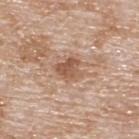Impression: Recorded during total-body skin imaging; not selected for excision or biopsy. Context: Located on the upper back. The subject is a male in their 80s. Automated image analysis of the tile measured border irregularity of about 3.5 on a 0–10 scale, internal color variation of about 3.5 on a 0–10 scale, and a peripheral color-asymmetry measure near 1. The software also gave a nevus-likeness score of about 0/100. About 3 mm across. Imaged with white-light lighting. A close-up tile cropped from a whole-body skin photograph, about 15 mm across.